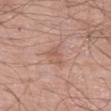Q: Is there a histopathology result?
A: total-body-photography surveillance lesion; no biopsy
Q: What is the anatomic site?
A: the left thigh
Q: What lighting was used for the tile?
A: white-light illumination
Q: What are the patient's age and sex?
A: male, aged around 70
Q: Lesion size?
A: ~3 mm (longest diameter)
Q: How was this image acquired?
A: ~15 mm tile from a whole-body skin photo